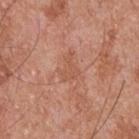Assessment:
The lesion was photographed on a routine skin check and not biopsied; there is no pathology result.
Image and clinical context:
A male patient aged approximately 55. A 15 mm close-up tile from a total-body photography series done for melanoma screening. Automated tile analysis of the lesion measured a lesion area of about 4.5 mm². The software also gave a mean CIELAB color near L≈55 a*≈24 b*≈32, about 6 CIELAB-L* units darker than the surrounding skin, and a normalized lesion–skin contrast near 4.5. It also reported a nevus-likeness score of about 0/100. From the upper back. Longest diameter approximately 3 mm.